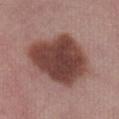* biopsy status · catalogued during a skin exam; not biopsied
* tile lighting · white-light illumination
* image source · ~15 mm tile from a whole-body skin photo
* body site · the abdomen
* subject · female, in their mid- to late 50s